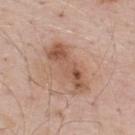notes=imaged on a skin check; not biopsied
site=the upper back
subject=male, aged around 70
diameter=≈6.5 mm
imaging modality=~15 mm tile from a whole-body skin photo
tile lighting=white-light